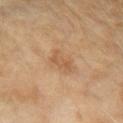The lesion's longest dimension is about 2.5 mm. From the left forearm. A region of skin cropped from a whole-body photographic capture, roughly 15 mm wide. This is a cross-polarized tile. A female subject about 60 years old.